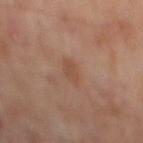Impression:
This lesion was catalogued during total-body skin photography and was not selected for biopsy.
Clinical summary:
Located on the mid back. The lesion-visualizer software estimated an area of roughly 4 mm², an eccentricity of roughly 0.9, and a shape-asymmetry score of about 0.15 (0 = symmetric). The analysis additionally found border irregularity of about 2 on a 0–10 scale and a peripheral color-asymmetry measure near 0.5. Cropped from a whole-body photographic skin survey; the tile spans about 15 mm. A male subject, aged 63 to 67.